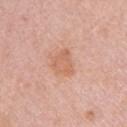Impression: This lesion was catalogued during total-body skin photography and was not selected for biopsy. Clinical summary: A roughly 15 mm field-of-view crop from a total-body skin photograph. Measured at roughly 3 mm in maximum diameter. This is a white-light tile. The lesion is on the left upper arm. The patient is a female aged 28 to 32. Automated image analysis of the tile measured an outline eccentricity of about 0.55 (0 = round, 1 = elongated) and a shape-asymmetry score of about 0.35 (0 = symmetric).